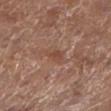Assessment:
The lesion was tiled from a total-body skin photograph and was not biopsied.
Context:
The tile uses white-light illumination. From the left lower leg. Cropped from a total-body skin-imaging series; the visible field is about 15 mm. The patient is a female aged 78 to 82.